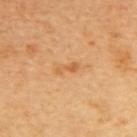Part of a total-body skin-imaging series; this lesion was reviewed on a skin check and was not flagged for biopsy. On the upper back. Longest diameter approximately 2.5 mm. A female subject aged 43 to 47. A roughly 15 mm field-of-view crop from a total-body skin photograph.